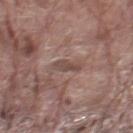Assessment: Captured during whole-body skin photography for melanoma surveillance; the lesion was not biopsied. Image and clinical context: Cropped from a total-body skin-imaging series; the visible field is about 15 mm. A male patient roughly 80 years of age. Located on the leg.